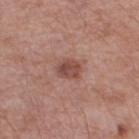{
  "lighting": "white-light",
  "patient": {
    "sex": "male",
    "age_approx": 55
  },
  "site": "left lower leg",
  "image": {
    "source": "total-body photography crop",
    "field_of_view_mm": 15
  },
  "lesion_size": {
    "long_diameter_mm_approx": 2.5
  }
}